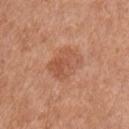biopsy status — no biopsy performed (imaged during a skin exam)
diameter — about 4 mm
anatomic site — the chest
subject — male, about 55 years old
image source — ~15 mm tile from a whole-body skin photo
lighting — white-light illumination
automated metrics — an area of roughly 12 mm², an outline eccentricity of about 0.5 (0 = round, 1 = elongated), and a symmetry-axis asymmetry near 0.15; a peripheral color-asymmetry measure near 1.5